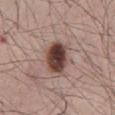{"biopsy_status": "not biopsied; imaged during a skin examination", "image": {"source": "total-body photography crop", "field_of_view_mm": 15}, "site": "abdomen", "patient": {"sex": "male", "age_approx": 60}}